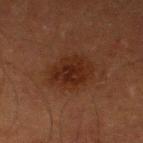Findings:
– follow-up · no biopsy performed (imaged during a skin exam)
– subject · male, in their mid-60s
– site · the leg
– lesion diameter · ≈5.5 mm
– imaging modality · total-body-photography crop, ~15 mm field of view